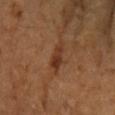Impression: The lesion was photographed on a routine skin check and not biopsied; there is no pathology result. Context: This is a cross-polarized tile. Located on the arm. Cropped from a whole-body photographic skin survey; the tile spans about 15 mm. A female patient, roughly 70 years of age. Approximately 4 mm at its widest. An algorithmic analysis of the crop reported a color-variation rating of about 3/10 and peripheral color asymmetry of about 1. It also reported a lesion-detection confidence of about 100/100.